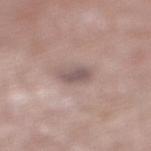notes: catalogued during a skin exam; not biopsied
illumination: white-light illumination
size: ≈2.5 mm
subject: male, aged around 80
location: the left leg
imaging modality: ~15 mm tile from a whole-body skin photo